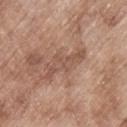Clinical impression: This lesion was catalogued during total-body skin photography and was not selected for biopsy. Image and clinical context: Imaged with white-light lighting. On the upper back. Measured at roughly 5.5 mm in maximum diameter. Cropped from a whole-body photographic skin survey; the tile spans about 15 mm. Automated tile analysis of the lesion measured a lesion area of about 9 mm², an outline eccentricity of about 0.9 (0 = round, 1 = elongated), and a shape-asymmetry score of about 0.45 (0 = symmetric). A male subject, in their mid-60s.